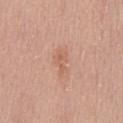  biopsy_status: not biopsied; imaged during a skin examination
  patient:
    sex: male
    age_approx: 50
  lighting: white-light
  automated_metrics:
    eccentricity: 0.9
    shape_asymmetry: 0.4
    cielab_L: 60
    cielab_a: 23
    cielab_b: 30
    vs_skin_darker_L: 7.0
    vs_skin_contrast_norm: 5.0
    border_irregularity_0_10: 4.5
    color_variation_0_10: 1.5
    peripheral_color_asymmetry: 0.5
  image:
    source: total-body photography crop
    field_of_view_mm: 15
  site: front of the torso
  lesion_size:
    long_diameter_mm_approx: 3.5